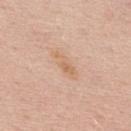Q: Was this lesion biopsied?
A: no biopsy performed (imaged during a skin exam)
Q: How was this image acquired?
A: total-body-photography crop, ~15 mm field of view
Q: Lesion location?
A: the back
Q: Patient demographics?
A: male, aged approximately 60